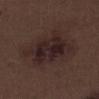The lesion was photographed on a routine skin check and not biopsied; there is no pathology result.
Automated image analysis of the tile measured a shape eccentricity near 0.7 and a symmetry-axis asymmetry near 0.2. It also reported a mean CIELAB color near L≈23 a*≈15 b*≈15, a lesion–skin lightness drop of about 7, and a lesion-to-skin contrast of about 9.5 (normalized; higher = more distinct). It also reported a classifier nevus-likeness of about 10/100.
The lesion is on the right lower leg.
A male subject, aged 68–72.
About 6.5 mm across.
A close-up tile cropped from a whole-body skin photograph, about 15 mm across.
This is a white-light tile.